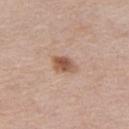  image:
    source: total-body photography crop
    field_of_view_mm: 15
  automated_metrics:
    area_mm2_approx: 5.0
    eccentricity: 0.75
    shape_asymmetry: 0.25
    cielab_L: 55
    cielab_a: 20
    cielab_b: 29
    vs_skin_darker_L: 13.0
    vs_skin_contrast_norm: 8.5
  lighting: white-light
  site: leg
  lesion_size:
    long_diameter_mm_approx: 3.0
  patient:
    sex: female
    age_approx: 40